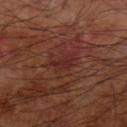biopsy status: total-body-photography surveillance lesion; no biopsy
location: the arm
automated lesion analysis: an area of roughly 4.5 mm², an eccentricity of roughly 0.85, and a shape-asymmetry score of about 0.4 (0 = symmetric); border irregularity of about 4.5 on a 0–10 scale, internal color variation of about 2 on a 0–10 scale, and radial color variation of about 0.5; lesion-presence confidence of about 100/100
tile lighting: cross-polarized
imaging modality: ~15 mm tile from a whole-body skin photo
lesion size: ≈3 mm
patient: male, in their 60s